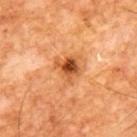- biopsy status — catalogued during a skin exam; not biopsied
- diameter — ≈3 mm
- illumination — cross-polarized illumination
- imaging modality — ~15 mm crop, total-body skin-cancer survey
- subject — male, aged 63 to 67
- automated lesion analysis — a color-variation rating of about 8/10 and peripheral color asymmetry of about 2.5; a nevus-likeness score of about 50/100 and a detector confidence of about 100 out of 100 that the crop contains a lesion